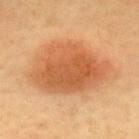The lesion was photographed on a routine skin check and not biopsied; there is no pathology result.
The patient is a male approximately 55 years of age.
A close-up tile cropped from a whole-body skin photograph, about 15 mm across.
On the upper back.
The lesion's longest dimension is about 9 mm.
Imaged with cross-polarized lighting.